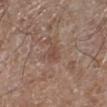The lesion was tiled from a total-body skin photograph and was not biopsied. The lesion is located on the left lower leg. Captured under white-light illumination. The subject is a male in their mid-60s. Cropped from a total-body skin-imaging series; the visible field is about 15 mm. Longest diameter approximately 2.5 mm.